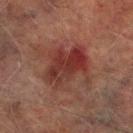Part of a total-body skin-imaging series; this lesion was reviewed on a skin check and was not flagged for biopsy. A 15 mm close-up extracted from a 3D total-body photography capture. Longest diameter approximately 5.5 mm. The total-body-photography lesion software estimated an area of roughly 19 mm², an eccentricity of roughly 0.6, and a shape-asymmetry score of about 0.4 (0 = symmetric). The software also gave internal color variation of about 7 on a 0–10 scale and radial color variation of about 2.5. The analysis additionally found an automated nevus-likeness rating near 0 out of 100 and a detector confidence of about 100 out of 100 that the crop contains a lesion. The lesion is located on the right lower leg. A male subject, in their mid-70s.